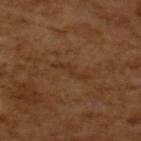Clinical impression:
The lesion was photographed on a routine skin check and not biopsied; there is no pathology result.